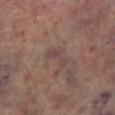Captured during whole-body skin photography for melanoma surveillance; the lesion was not biopsied. This image is a 15 mm lesion crop taken from a total-body photograph. From the right lower leg. Longest diameter approximately 3.5 mm. Automated tile analysis of the lesion measured a classifier nevus-likeness of about 0/100 and a detector confidence of about 95 out of 100 that the crop contains a lesion. A male subject, in their 70s. The tile uses white-light illumination.